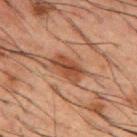Captured during whole-body skin photography for melanoma surveillance; the lesion was not biopsied. Located on the mid back. A 15 mm close-up extracted from a 3D total-body photography capture. The total-body-photography lesion software estimated a footprint of about 6.5 mm², an eccentricity of roughly 0.85, and two-axis asymmetry of about 0.25. The analysis additionally found about 9 CIELAB-L* units darker than the surrounding skin and a lesion-to-skin contrast of about 8.5 (normalized; higher = more distinct). The analysis additionally found a border-irregularity rating of about 2.5/10, internal color variation of about 3.5 on a 0–10 scale, and radial color variation of about 1.5. The subject is a male in their 50s. This is a cross-polarized tile. About 4 mm across.